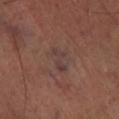Captured under cross-polarized illumination. On the right lower leg. The lesion's longest dimension is about 3.5 mm. The total-body-photography lesion software estimated a border-irregularity index near 5.5/10, internal color variation of about 0 on a 0–10 scale, and peripheral color asymmetry of about 0. A close-up tile cropped from a whole-body skin photograph, about 15 mm across. A male subject aged 68 to 72.